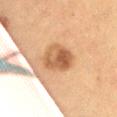follow-up — total-body-photography surveillance lesion; no biopsy
lighting — cross-polarized
lesion diameter — ~4 mm (longest diameter)
patient — female, roughly 50 years of age
image source — 15 mm crop, total-body photography
body site — the abdomen